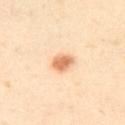| feature | finding |
|---|---|
| follow-up | imaged on a skin check; not biopsied |
| subject | female, roughly 40 years of age |
| image source | total-body-photography crop, ~15 mm field of view |
| anatomic site | the left upper arm |
| size | ~3 mm (longest diameter) |
| illumination | cross-polarized |